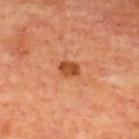Assessment: The lesion was photographed on a routine skin check and not biopsied; there is no pathology result. Clinical summary: Automated image analysis of the tile measured a detector confidence of about 100 out of 100 that the crop contains a lesion. Imaged with cross-polarized lighting. Located on the upper back. A 15 mm crop from a total-body photograph taken for skin-cancer surveillance. The patient is aged 63 to 67. The lesion's longest dimension is about 2.5 mm.